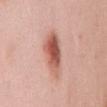Captured during whole-body skin photography for melanoma surveillance; the lesion was not biopsied.
Cropped from a total-body skin-imaging series; the visible field is about 15 mm.
A female patient, about 65 years old.
Imaged with white-light lighting.
From the mid back.
Measured at roughly 6 mm in maximum diameter.